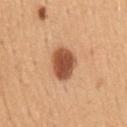Q: Was a biopsy performed?
A: imaged on a skin check; not biopsied
Q: Who is the patient?
A: male, approximately 50 years of age
Q: How large is the lesion?
A: ~4 mm (longest diameter)
Q: Lesion location?
A: the mid back
Q: What lighting was used for the tile?
A: white-light illumination
Q: Automated lesion metrics?
A: a lesion color around L≈52 a*≈26 b*≈35 in CIELAB and a lesion-to-skin contrast of about 11.5 (normalized; higher = more distinct); a classifier nevus-likeness of about 100/100 and a detector confidence of about 100 out of 100 that the crop contains a lesion
Q: How was this image acquired?
A: 15 mm crop, total-body photography